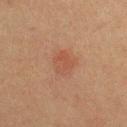Q: Is there a histopathology result?
A: total-body-photography surveillance lesion; no biopsy
Q: How was the tile lit?
A: cross-polarized
Q: Who is the patient?
A: male, approximately 40 years of age
Q: What kind of image is this?
A: total-body-photography crop, ~15 mm field of view
Q: What is the anatomic site?
A: the chest
Q: How large is the lesion?
A: ≈3 mm